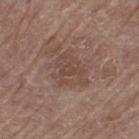– follow-up: total-body-photography surveillance lesion; no biopsy